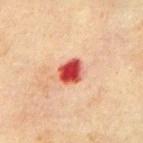{"biopsy_status": "not biopsied; imaged during a skin examination", "lighting": "cross-polarized", "automated_metrics": {"nevus_likeness_0_100": 0, "lesion_detection_confidence_0_100": 100}, "image": {"source": "total-body photography crop", "field_of_view_mm": 15}, "lesion_size": {"long_diameter_mm_approx": 3.0}, "patient": {"sex": "male", "age_approx": 70}, "site": "abdomen"}